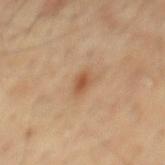Q: Was a biopsy performed?
A: imaged on a skin check; not biopsied
Q: What is the anatomic site?
A: the mid back
Q: What are the patient's age and sex?
A: male, in their mid- to late 50s
Q: What is the imaging modality?
A: ~15 mm tile from a whole-body skin photo